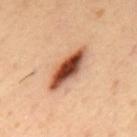Impression:
The lesion was tiled from a total-body skin photograph and was not biopsied.
Background:
Located on the mid back. Captured under cross-polarized illumination. Cropped from a whole-body photographic skin survey; the tile spans about 15 mm. Measured at roughly 6 mm in maximum diameter. A male subject aged around 55.